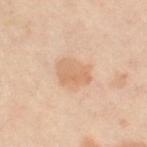The lesion was photographed on a routine skin check and not biopsied; there is no pathology result.
The lesion's longest dimension is about 4 mm.
The patient is a female aged 48–52.
Imaged with cross-polarized lighting.
The lesion is located on the right thigh.
This image is a 15 mm lesion crop taken from a total-body photograph.
The total-body-photography lesion software estimated a lesion area of about 9.5 mm², an outline eccentricity of about 0.65 (0 = round, 1 = elongated), and a symmetry-axis asymmetry near 0.2. The software also gave a classifier nevus-likeness of about 20/100 and a lesion-detection confidence of about 100/100.